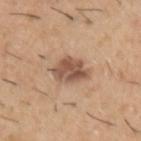This lesion was catalogued during total-body skin photography and was not selected for biopsy.
A 15 mm crop from a total-body photograph taken for skin-cancer surveillance.
The lesion-visualizer software estimated an area of roughly 10 mm², a shape eccentricity near 0.8, and a shape-asymmetry score of about 0.2 (0 = symmetric). It also reported a classifier nevus-likeness of about 70/100 and lesion-presence confidence of about 100/100.
The lesion is on the left upper arm.
A male patient, about 40 years old.